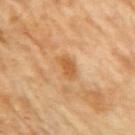Findings:
• workup — catalogued during a skin exam; not biopsied
• body site — the right upper arm
• patient — female, in their mid-50s
• illumination — cross-polarized illumination
• imaging modality — total-body-photography crop, ~15 mm field of view